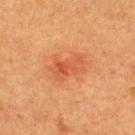{
  "biopsy_status": "not biopsied; imaged during a skin examination",
  "patient": {
    "sex": "male",
    "age_approx": 40
  },
  "lesion_size": {
    "long_diameter_mm_approx": 4.0
  },
  "automated_metrics": {
    "cielab_L": 46,
    "cielab_a": 28,
    "cielab_b": 35,
    "vs_skin_darker_L": 7.0,
    "vs_skin_contrast_norm": 5.5,
    "border_irregularity_0_10": 3.5,
    "peripheral_color_asymmetry": 1.5
  },
  "site": "upper back",
  "image": {
    "source": "total-body photography crop",
    "field_of_view_mm": 15
  }
}